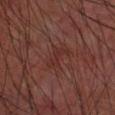The lesion was photographed on a routine skin check and not biopsied; there is no pathology result. A male subject, aged around 60. A lesion tile, about 15 mm wide, cut from a 3D total-body photograph. Longest diameter approximately 3.5 mm. Imaged with cross-polarized lighting. On the right forearm.